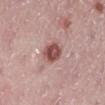follow-up: catalogued during a skin exam; not biopsied
size: about 3.5 mm
subject: female, aged 23 to 27
site: the left lower leg
imaging modality: ~15 mm tile from a whole-body skin photo